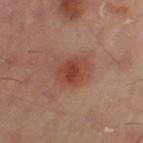workup: no biopsy performed (imaged during a skin exam) | lesion diameter: ≈4 mm | image-analysis metrics: border irregularity of about 2.5 on a 0–10 scale, a within-lesion color-variation index near 4.5/10, and radial color variation of about 1; a classifier nevus-likeness of about 95/100 and a detector confidence of about 100 out of 100 that the crop contains a lesion | anatomic site: the left thigh | lighting: cross-polarized illumination | image: 15 mm crop, total-body photography.